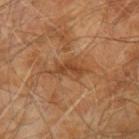Recorded during total-body skin imaging; not selected for excision or biopsy. The tile uses cross-polarized illumination. A male patient aged 68–72. An algorithmic analysis of the crop reported an average lesion color of about L≈43 a*≈23 b*≈35 (CIELAB), roughly 9 lightness units darker than nearby skin, and a normalized lesion–skin contrast near 7. On the left upper arm. A roughly 15 mm field-of-view crop from a total-body skin photograph. Approximately 4 mm at its widest.